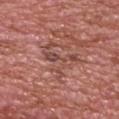{
  "biopsy_status": "not biopsied; imaged during a skin examination",
  "image": {
    "source": "total-body photography crop",
    "field_of_view_mm": 15
  },
  "lighting": "white-light",
  "automated_metrics": {
    "vs_skin_darker_L": 8.0
  },
  "patient": {
    "sex": "male",
    "age_approx": 70
  },
  "lesion_size": {
    "long_diameter_mm_approx": 5.5
  },
  "site": "upper back"
}